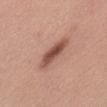Captured during whole-body skin photography for melanoma surveillance; the lesion was not biopsied.
A female subject, aged 48–52.
Captured under white-light illumination.
Automated tile analysis of the lesion measured a shape eccentricity near 0.95 and two-axis asymmetry of about 0.25. And it measured a border-irregularity rating of about 3.5/10, internal color variation of about 4.5 on a 0–10 scale, and radial color variation of about 1. The software also gave an automated nevus-likeness rating near 55 out of 100 and lesion-presence confidence of about 100/100.
Located on the abdomen.
A 15 mm close-up extracted from a 3D total-body photography capture.